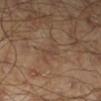The lesion was photographed on a routine skin check and not biopsied; there is no pathology result.